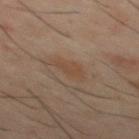- biopsy status · imaged on a skin check; not biopsied
- site · the mid back
- size · ~3.5 mm (longest diameter)
- lighting · cross-polarized
- patient · male, aged 38 to 42
- image · total-body-photography crop, ~15 mm field of view